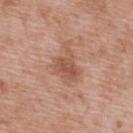Captured during whole-body skin photography for melanoma surveillance; the lesion was not biopsied. The patient is a male aged around 50. Automated tile analysis of the lesion measured an average lesion color of about L≈54 a*≈23 b*≈29 (CIELAB), a lesion–skin lightness drop of about 9, and a lesion-to-skin contrast of about 6.5 (normalized; higher = more distinct). The software also gave a border-irregularity rating of about 5.5/10, a within-lesion color-variation index near 3.5/10, and peripheral color asymmetry of about 1.5. A 15 mm crop from a total-body photograph taken for skin-cancer surveillance. The recorded lesion diameter is about 4.5 mm. The lesion is located on the back.